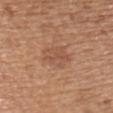  biopsy_status: not biopsied; imaged during a skin examination